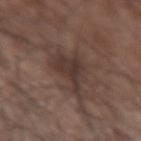Recorded during total-body skin imaging; not selected for excision or biopsy. The lesion is located on the left upper arm. This image is a 15 mm lesion crop taken from a total-body photograph. This is a white-light tile. A male subject, approximately 65 years of age. The lesion's longest dimension is about 5 mm. The lesion-visualizer software estimated an eccentricity of roughly 0.65 and two-axis asymmetry of about 0.5. It also reported a lesion color around L≈35 a*≈14 b*≈20 in CIELAB, about 8 CIELAB-L* units darker than the surrounding skin, and a lesion-to-skin contrast of about 7.5 (normalized; higher = more distinct). The analysis additionally found a border-irregularity rating of about 6/10, a color-variation rating of about 3.5/10, and radial color variation of about 1.